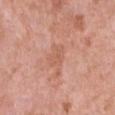<tbp_lesion>
<biopsy_status>not biopsied; imaged during a skin examination</biopsy_status>
<lighting>white-light</lighting>
<site>chest</site>
<lesion_size>
  <long_diameter_mm_approx>2.5</long_diameter_mm_approx>
</lesion_size>
<image>
  <source>total-body photography crop</source>
  <field_of_view_mm>15</field_of_view_mm>
</image>
<patient>
  <sex>female</sex>
  <age_approx>50</age_approx>
</patient>
</tbp_lesion>